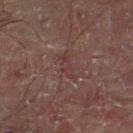follow-up: imaged on a skin check; not biopsied
patient: male, in their 60s
illumination: cross-polarized illumination
automated lesion analysis: a footprint of about 3 mm² and two-axis asymmetry of about 0.55; a mean CIELAB color near L≈32 a*≈18 b*≈16 and a lesion-to-skin contrast of about 5 (normalized; higher = more distinct)
body site: the leg
acquisition: ~15 mm crop, total-body skin-cancer survey